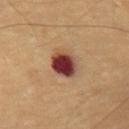The lesion was tiled from a total-body skin photograph and was not biopsied. Approximately 4 mm at its widest. A 15 mm crop from a total-body photograph taken for skin-cancer surveillance. A male subject, in their mid- to late 60s. The tile uses cross-polarized illumination. On the back.